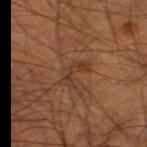An algorithmic analysis of the crop reported a lesion–skin lightness drop of about 5. The software also gave border irregularity of about 7.5 on a 0–10 scale and a peripheral color-asymmetry measure near 0. Located on the left thigh. A male subject, aged 48 to 52. A 15 mm crop from a total-body photograph taken for skin-cancer surveillance. Imaged with cross-polarized lighting. About 4 mm across.